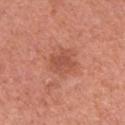Imaged during a routine full-body skin examination; the lesion was not biopsied and no histopathology is available. Cropped from a total-body skin-imaging series; the visible field is about 15 mm. This is a white-light tile. The lesion is located on the left upper arm. A female subject, in their 50s. The recorded lesion diameter is about 3.5 mm.